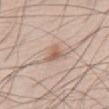Clinical impression: Recorded during total-body skin imaging; not selected for excision or biopsy. Background: The tile uses white-light illumination. Approximately 3 mm at its widest. The lesion is located on the abdomen. The lesion-visualizer software estimated a classifier nevus-likeness of about 65/100 and a detector confidence of about 100 out of 100 that the crop contains a lesion. A close-up tile cropped from a whole-body skin photograph, about 15 mm across. A male subject roughly 60 years of age.